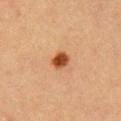Clinical impression:
Captured during whole-body skin photography for melanoma surveillance; the lesion was not biopsied.
Acquisition and patient details:
A male subject, in their 40s. A lesion tile, about 15 mm wide, cut from a 3D total-body photograph. On the chest.